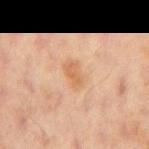Imaged during a routine full-body skin examination; the lesion was not biopsied and no histopathology is available. A lesion tile, about 15 mm wide, cut from a 3D total-body photograph. Located on the mid back. The lesion's longest dimension is about 3.5 mm. The patient is a male aged around 60. Captured under cross-polarized illumination.